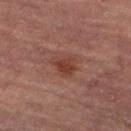Captured during whole-body skin photography for melanoma surveillance; the lesion was not biopsied.
Longest diameter approximately 3 mm.
Located on the leg.
The subject is a female aged 68 to 72.
A 15 mm close-up extracted from a 3D total-body photography capture.
This is a cross-polarized tile.